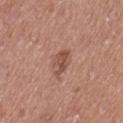  biopsy_status: not biopsied; imaged during a skin examination
  patient:
    sex: female
    age_approx: 40
  lighting: white-light
  image:
    source: total-body photography crop
    field_of_view_mm: 15
  site: leg
  automated_metrics:
    area_mm2_approx: 5.0
    eccentricity: 0.85
    cielab_L: 50
    cielab_a: 22
    cielab_b: 27
    vs_skin_darker_L: 10.0
    border_irregularity_0_10: 3.5
    peripheral_color_asymmetry: 1.0